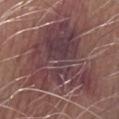Imaged during a routine full-body skin examination; the lesion was not biopsied and no histopathology is available. Captured under white-light illumination. A close-up tile cropped from a whole-body skin photograph, about 15 mm across. The subject is a male aged around 65. From the left forearm. Measured at roughly 10.5 mm in maximum diameter. Automated image analysis of the tile measured an area of roughly 60 mm², a shape eccentricity near 0.45, and a symmetry-axis asymmetry near 0.5. And it measured a lesion–skin lightness drop of about 10 and a normalized lesion–skin contrast near 9.5. The analysis additionally found a border-irregularity index near 8.5/10, a color-variation rating of about 7/10, and a peripheral color-asymmetry measure near 2.5. The software also gave a nevus-likeness score of about 0/100 and lesion-presence confidence of about 85/100.